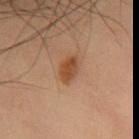follow-up — total-body-photography surveillance lesion; no biopsy
illumination — cross-polarized
patient — male, aged 53 to 57
image source — 15 mm crop, total-body photography
lesion diameter — ≈3 mm
image-analysis metrics — a border-irregularity rating of about 1.5/10 and a peripheral color-asymmetry measure near 0.5
location — the chest